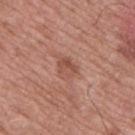{
  "biopsy_status": "not biopsied; imaged during a skin examination",
  "patient": {
    "sex": "male",
    "age_approx": 65
  },
  "automated_metrics": {
    "cielab_L": 51,
    "cielab_a": 24,
    "cielab_b": 28,
    "vs_skin_darker_L": 8.0,
    "vs_skin_contrast_norm": 6.0,
    "border_irregularity_0_10": 2.5,
    "color_variation_0_10": 3.5,
    "peripheral_color_asymmetry": 1.5
  },
  "image": {
    "source": "total-body photography crop",
    "field_of_view_mm": 15
  },
  "site": "upper back",
  "lighting": "white-light",
  "lesion_size": {
    "long_diameter_mm_approx": 2.5
  }
}